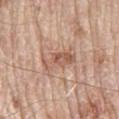workup: no biopsy performed (imaged during a skin exam).